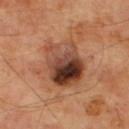A 15 mm crop from a total-body photograph taken for skin-cancer surveillance. Approximately 6.5 mm at its widest. A male patient, in their mid-60s. From the upper back. Histopathologically confirmed as a squamous cell carcinoma in situ, classified as a malignant lesion.